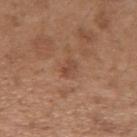Q: Was a biopsy performed?
A: imaged on a skin check; not biopsied
Q: What are the patient's age and sex?
A: female, aged 28 to 32
Q: How large is the lesion?
A: about 2.5 mm
Q: What is the imaging modality?
A: ~15 mm crop, total-body skin-cancer survey
Q: Where on the body is the lesion?
A: the left forearm
Q: What lighting was used for the tile?
A: white-light